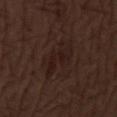Clinical impression:
The lesion was photographed on a routine skin check and not biopsied; there is no pathology result.
Background:
A close-up tile cropped from a whole-body skin photograph, about 15 mm across. Measured at roughly 5.5 mm in maximum diameter. The lesion is on the leg. An algorithmic analysis of the crop reported an area of roughly 11 mm², an outline eccentricity of about 0.85 (0 = round, 1 = elongated), and a shape-asymmetry score of about 0.4 (0 = symmetric). The analysis additionally found an automated nevus-likeness rating near 0 out of 100 and a lesion-detection confidence of about 100/100. A male patient aged 68 to 72.